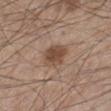Q: Was a biopsy performed?
A: total-body-photography surveillance lesion; no biopsy
Q: What did automated image analysis measure?
A: a classifier nevus-likeness of about 90/100
Q: What lighting was used for the tile?
A: white-light
Q: Patient demographics?
A: male, in their mid- to late 40s
Q: What is the lesion's diameter?
A: ≈3 mm
Q: Lesion location?
A: the leg
Q: How was this image acquired?
A: total-body-photography crop, ~15 mm field of view